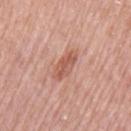Impression: Captured during whole-body skin photography for melanoma surveillance; the lesion was not biopsied. Context: An algorithmic analysis of the crop reported an average lesion color of about L≈57 a*≈24 b*≈30 (CIELAB), roughly 10 lightness units darker than nearby skin, and a normalized border contrast of about 6.5. The analysis additionally found a border-irregularity rating of about 2/10 and peripheral color asymmetry of about 1. The analysis additionally found an automated nevus-likeness rating near 30 out of 100 and a lesion-detection confidence of about 100/100. Imaged with white-light lighting. The lesion is on the right upper arm. A 15 mm crop from a total-body photograph taken for skin-cancer surveillance. A female subject, approximately 65 years of age.